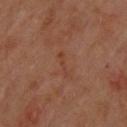Clinical impression: This lesion was catalogued during total-body skin photography and was not selected for biopsy. Background: A male subject about 65 years old. A region of skin cropped from a whole-body photographic capture, roughly 15 mm wide. Approximately 3 mm at its widest. On the upper back.